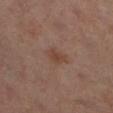Impression: Recorded during total-body skin imaging; not selected for excision or biopsy. Clinical summary: Located on the left lower leg. A female patient, approximately 60 years of age. This is a cross-polarized tile. A 15 mm close-up tile from a total-body photography series done for melanoma screening.